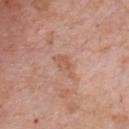{"biopsy_status": "not biopsied; imaged during a skin examination", "image": {"source": "total-body photography crop", "field_of_view_mm": 15}, "automated_metrics": {"area_mm2_approx": 3.5, "eccentricity": 0.85, "shape_asymmetry": 0.4, "border_irregularity_0_10": 4.5, "nevus_likeness_0_100": 0, "lesion_detection_confidence_0_100": 100}, "site": "chest", "lesion_size": {"long_diameter_mm_approx": 3.0}, "patient": {"sex": "male", "age_approx": 60}}